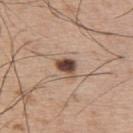Q: Was a biopsy performed?
A: catalogued during a skin exam; not biopsied
Q: How large is the lesion?
A: ~3 mm (longest diameter)
Q: How was this image acquired?
A: 15 mm crop, total-body photography
Q: Lesion location?
A: the upper back
Q: How was the tile lit?
A: white-light illumination
Q: Automated lesion metrics?
A: a footprint of about 4.5 mm² and a symmetry-axis asymmetry near 0.3; an average lesion color of about L≈45 a*≈18 b*≈25 (CIELAB), roughly 19 lightness units darker than nearby skin, and a normalized border contrast of about 13
Q: Who is the patient?
A: male, aged approximately 50